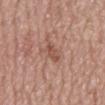Findings:
• TBP lesion metrics — a border-irregularity index near 4/10 and internal color variation of about 0 on a 0–10 scale
• lighting — white-light illumination
• image source — total-body-photography crop, ~15 mm field of view
• diameter — about 3 mm
• subject — male, aged around 80
• body site — the mid back